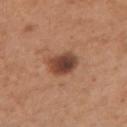Q: Was a biopsy performed?
A: no biopsy performed (imaged during a skin exam)
Q: How was this image acquired?
A: 15 mm crop, total-body photography
Q: Patient demographics?
A: female, aged around 30
Q: What lighting was used for the tile?
A: white-light illumination
Q: Lesion location?
A: the left upper arm